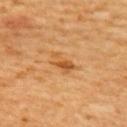Recorded during total-body skin imaging; not selected for excision or biopsy.
A roughly 15 mm field-of-view crop from a total-body skin photograph.
Longest diameter approximately 3.5 mm.
On the upper back.
Automated image analysis of the tile measured a lesion color around L≈55 a*≈24 b*≈44 in CIELAB, a lesion–skin lightness drop of about 9, and a normalized border contrast of about 6.5. It also reported a nevus-likeness score of about 60/100 and lesion-presence confidence of about 100/100.
Imaged with cross-polarized lighting.
The subject is a female about 65 years old.